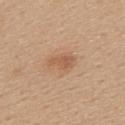This image is a 15 mm lesion crop taken from a total-body photograph.
Located on the upper back.
The patient is a female approximately 45 years of age.
The tile uses white-light illumination.
The lesion's longest dimension is about 3 mm.